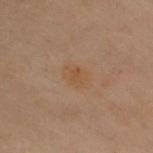Assessment:
This lesion was catalogued during total-body skin photography and was not selected for biopsy.
Context:
A roughly 15 mm field-of-view crop from a total-body skin photograph. Approximately 3.5 mm at its widest. The patient is a female aged approximately 60. The tile uses cross-polarized illumination. Located on the upper back.